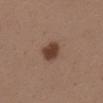The lesion is located on the upper back. A lesion tile, about 15 mm wide, cut from a 3D total-body photograph. A male patient, aged 38–42.